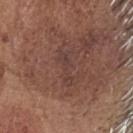Case summary:
– notes — total-body-photography surveillance lesion; no biopsy
– size — ≈4.5 mm
– lighting — white-light
– imaging modality — ~15 mm tile from a whole-body skin photo
– site — the head or neck
– subject — male, in their mid-70s